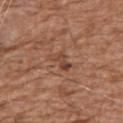- follow-up: no biopsy performed (imaged during a skin exam)
- lesion diameter: ≈3 mm
- imaging modality: 15 mm crop, total-body photography
- patient: male, approximately 75 years of age
- body site: the arm
- illumination: white-light illumination